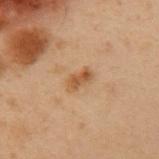biopsy status = no biopsy performed (imaged during a skin exam)
body site = the left upper arm
patient = male, roughly 55 years of age
lighting = cross-polarized illumination
diameter = about 3 mm
image source = 15 mm crop, total-body photography